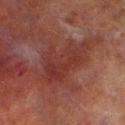automated_metrics:
  vs_skin_darker_L: 6.0
  border_irregularity_0_10: 6.0
  color_variation_0_10: 3.5
  peripheral_color_asymmetry: 1.0
  nevus_likeness_0_100: 0
  lesion_detection_confidence_0_100: 90
patient:
  sex: male
  age_approx: 70
site: left lower leg
image:
  source: total-body photography crop
  field_of_view_mm: 15
lesion_size:
  long_diameter_mm_approx: 7.5